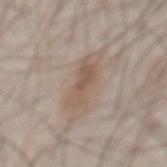Approximately 5 mm at its widest.
A male subject aged around 55.
This is a white-light tile.
A close-up tile cropped from a whole-body skin photograph, about 15 mm across.
The lesion is located on the chest.
Automated tile analysis of the lesion measured an area of roughly 8 mm². It also reported a color-variation rating of about 3.5/10 and a peripheral color-asymmetry measure near 1. The analysis additionally found a classifier nevus-likeness of about 75/100 and a detector confidence of about 100 out of 100 that the crop contains a lesion.